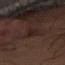Clinical impression:
Imaged during a routine full-body skin examination; the lesion was not biopsied and no histopathology is available.
Background:
Longest diameter approximately 3.5 mm. A female subject roughly 40 years of age. This image is a 15 mm lesion crop taken from a total-body photograph. The total-body-photography lesion software estimated a lesion color around L≈22 a*≈15 b*≈17 in CIELAB, a lesion–skin lightness drop of about 5, and a normalized lesion–skin contrast near 6.5. This is a cross-polarized tile. The lesion is on the left leg.